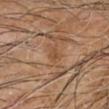Findings:
• location · the arm
• image · ~15 mm crop, total-body skin-cancer survey
• TBP lesion metrics · a lesion area of about 3 mm² and a shape eccentricity near 0.85; a mean CIELAB color near L≈49 a*≈20 b*≈33, about 6 CIELAB-L* units darker than the surrounding skin, and a normalized lesion–skin contrast near 5.5; a border-irregularity rating of about 3.5/10, a color-variation rating of about 1/10, and peripheral color asymmetry of about 0.5
• lesion diameter · about 3 mm
• patient · male, aged 63–67